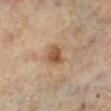biopsy status=total-body-photography surveillance lesion; no biopsy